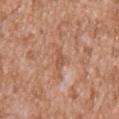Impression:
Captured during whole-body skin photography for melanoma surveillance; the lesion was not biopsied.